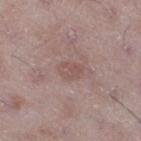The lesion was tiled from a total-body skin photograph and was not biopsied.
The subject is a male in their 50s.
Automated tile analysis of the lesion measured a classifier nevus-likeness of about 0/100.
A 15 mm close-up extracted from a 3D total-body photography capture.
The tile uses white-light illumination.
Located on the leg.